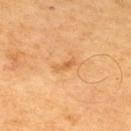acquisition: 15 mm crop, total-body photography | tile lighting: cross-polarized illumination | patient: male, in their mid-60s | lesion diameter: about 2.5 mm | automated lesion analysis: an eccentricity of roughly 0.9 and a shape-asymmetry score of about 0.35 (0 = symmetric); a lesion color around L≈62 a*≈24 b*≈45 in CIELAB, a lesion–skin lightness drop of about 8, and a lesion-to-skin contrast of about 5.5 (normalized; higher = more distinct); a border-irregularity rating of about 4/10, internal color variation of about 0 on a 0–10 scale, and a peripheral color-asymmetry measure near 0; an automated nevus-likeness rating near 0 out of 100 and a lesion-detection confidence of about 100/100.